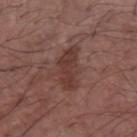Q: Was a biopsy performed?
A: total-body-photography surveillance lesion; no biopsy
Q: Where on the body is the lesion?
A: the arm
Q: How was this image acquired?
A: total-body-photography crop, ~15 mm field of view
Q: Lesion size?
A: ≈5 mm
Q: Illumination type?
A: white-light illumination
Q: What are the patient's age and sex?
A: male, approximately 70 years of age